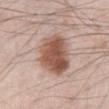Impression: Recorded during total-body skin imaging; not selected for excision or biopsy. Acquisition and patient details: About 5.5 mm across. A male patient, aged 68 to 72. A 15 mm close-up extracted from a 3D total-body photography capture. The lesion is located on the abdomen.